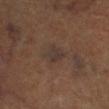Imaged during a routine full-body skin examination; the lesion was not biopsied and no histopathology is available. Cropped from a total-body skin-imaging series; the visible field is about 15 mm. Approximately 2.5 mm at its widest. The patient is a female aged approximately 65. Located on the left lower leg. This is a cross-polarized tile. The total-body-photography lesion software estimated an area of roughly 4.5 mm², a shape eccentricity near 0.65, and a shape-asymmetry score of about 0.3 (0 = symmetric). It also reported a classifier nevus-likeness of about 0/100.